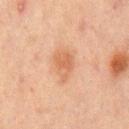The lesion was tiled from a total-body skin photograph and was not biopsied. A male subject, aged approximately 65. On the mid back. A close-up tile cropped from a whole-body skin photograph, about 15 mm across.